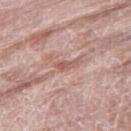{
  "biopsy_status": "not biopsied; imaged during a skin examination",
  "lesion_size": {
    "long_diameter_mm_approx": 2.5
  },
  "image": {
    "source": "total-body photography crop",
    "field_of_view_mm": 15
  },
  "patient": {
    "sex": "male",
    "age_approx": 80
  },
  "site": "right thigh",
  "lighting": "white-light",
  "automated_metrics": {
    "color_variation_0_10": 0.0,
    "peripheral_color_asymmetry": 0.0
  }
}